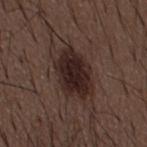Impression:
The lesion was photographed on a routine skin check and not biopsied; there is no pathology result.
Acquisition and patient details:
Cropped from a total-body skin-imaging series; the visible field is about 15 mm. A male subject, in their 50s. The recorded lesion diameter is about 11.5 mm. From the back. Imaged with white-light lighting.